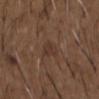| key | value |
|---|---|
| biopsy status | no biopsy performed (imaged during a skin exam) |
| diameter | about 2.5 mm |
| anatomic site | the chest |
| image | total-body-photography crop, ~15 mm field of view |
| illumination | white-light |
| subject | male, aged approximately 50 |
| TBP lesion metrics | a mean CIELAB color near L≈34 a*≈16 b*≈24 and a lesion–skin lightness drop of about 6; a border-irregularity rating of about 3/10 and a within-lesion color-variation index near 1.5/10; a nevus-likeness score of about 0/100 |